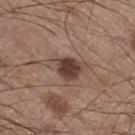<lesion>
<biopsy_status>not biopsied; imaged during a skin examination</biopsy_status>
<image>
  <source>total-body photography crop</source>
  <field_of_view_mm>15</field_of_view_mm>
</image>
<patient>
  <sex>male</sex>
  <age_approx>60</age_approx>
</patient>
<site>right thigh</site>
</lesion>